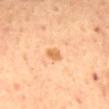<tbp_lesion>
  <biopsy_status>not biopsied; imaged during a skin examination</biopsy_status>
  <image>
    <source>total-body photography crop</source>
    <field_of_view_mm>15</field_of_view_mm>
  </image>
  <patient>
    <sex>male</sex>
    <age_approx>65</age_approx>
  </patient>
  <automated_metrics>
    <area_mm2_approx>2.5</area_mm2_approx>
    <cielab_L>68</cielab_L>
    <cielab_a>24</cielab_a>
    <cielab_b>44</cielab_b>
    <vs_skin_darker_L>10.0</vs_skin_darker_L>
    <vs_skin_contrast_norm>7.5</vs_skin_contrast_norm>
    <border_irregularity_0_10>2.5</border_irregularity_0_10>
    <color_variation_0_10>2.0</color_variation_0_10>
    <peripheral_color_asymmetry>1.0</peripheral_color_asymmetry>
  </automated_metrics>
  <lighting>cross-polarized</lighting>
  <site>mid back</site>
  <lesion_size>
    <long_diameter_mm_approx>2.0</long_diameter_mm_approx>
  </lesion_size>
</tbp_lesion>